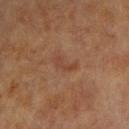Notes:
* patient · female, aged approximately 80
* body site · the left forearm
* imaging modality · 15 mm crop, total-body photography